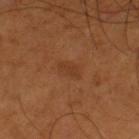Impression: Imaged during a routine full-body skin examination; the lesion was not biopsied and no histopathology is available. Background: About 2.5 mm across. Captured under cross-polarized illumination. The total-body-photography lesion software estimated a lesion-detection confidence of about 100/100. A 15 mm close-up tile from a total-body photography series done for melanoma screening. Located on the left thigh. A male patient, about 55 years old.